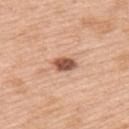{
  "site": "left upper arm",
  "image": {
    "source": "total-body photography crop",
    "field_of_view_mm": 15
  },
  "lighting": "white-light",
  "patient": {
    "sex": "male",
    "age_approx": 60
  }
}